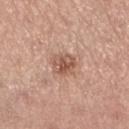The lesion was photographed on a routine skin check and not biopsied; there is no pathology result.
A close-up tile cropped from a whole-body skin photograph, about 15 mm across.
The total-body-photography lesion software estimated roughly 11 lightness units darker than nearby skin and a normalized lesion–skin contrast near 7.5. The analysis additionally found a lesion-detection confidence of about 100/100.
Located on the left lower leg.
The lesion's longest dimension is about 3.5 mm.
A female patient, about 65 years old.